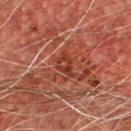Impression:
This lesion was catalogued during total-body skin photography and was not selected for biopsy.
Background:
The lesion is on the front of the torso. A 15 mm close-up tile from a total-body photography series done for melanoma screening. A male patient, aged 63 to 67.